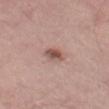Impression:
Imaged during a routine full-body skin examination; the lesion was not biopsied and no histopathology is available.
Image and clinical context:
The lesion is located on the left thigh. A 15 mm close-up extracted from a 3D total-body photography capture. About 2.5 mm across. Imaged with white-light lighting. A male subject in their 60s.